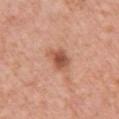{"image": {"source": "total-body photography crop", "field_of_view_mm": 15}, "patient": {"sex": "female", "age_approx": 40}, "site": "front of the torso"}